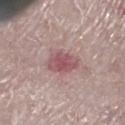Impression: This lesion was catalogued during total-body skin photography and was not selected for biopsy.